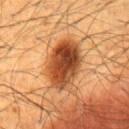biopsy_status: not biopsied; imaged during a skin examination
image:
  source: total-body photography crop
  field_of_view_mm: 15
lighting: cross-polarized
patient:
  sex: male
  age_approx: 60
site: chest
lesion_size:
  long_diameter_mm_approx: 6.0